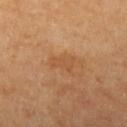Impression: No biopsy was performed on this lesion — it was imaged during a full skin examination and was not determined to be concerning. Context: A female patient, in their mid- to late 60s. Imaged with cross-polarized lighting. The lesion is on the left upper arm. The recorded lesion diameter is about 3 mm. Cropped from a whole-body photographic skin survey; the tile spans about 15 mm.